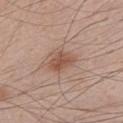{
  "biopsy_status": "not biopsied; imaged during a skin examination",
  "lighting": "white-light",
  "site": "front of the torso",
  "automated_metrics": {
    "cielab_L": 52,
    "cielab_a": 20,
    "cielab_b": 29,
    "vs_skin_darker_L": 10.0
  },
  "patient": {
    "sex": "male",
    "age_approx": 60
  },
  "lesion_size": {
    "long_diameter_mm_approx": 3.5
  },
  "image": {
    "source": "total-body photography crop",
    "field_of_view_mm": 15
  }
}